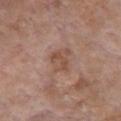The lesion was photographed on a routine skin check and not biopsied; there is no pathology result. The lesion-visualizer software estimated a mean CIELAB color near L≈50 a*≈19 b*≈27, about 8 CIELAB-L* units darker than the surrounding skin, and a normalized lesion–skin contrast near 6. It also reported a border-irregularity index near 4/10, a within-lesion color-variation index near 3/10, and a peripheral color-asymmetry measure near 1. The analysis additionally found an automated nevus-likeness rating near 0 out of 100 and lesion-presence confidence of about 100/100. A region of skin cropped from a whole-body photographic capture, roughly 15 mm wide. The patient is a female aged approximately 85 or older. The tile uses white-light illumination. The lesion is on the chest. Longest diameter approximately 3 mm.